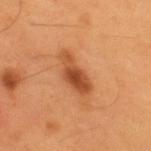Recorded during total-body skin imaging; not selected for excision or biopsy. About 5 mm across. A 15 mm close-up extracted from a 3D total-body photography capture. A male patient aged 53 to 57. The lesion-visualizer software estimated an area of roughly 8 mm² and two-axis asymmetry of about 0.3. The software also gave a lesion color around L≈48 a*≈28 b*≈39 in CIELAB, a lesion–skin lightness drop of about 13, and a normalized lesion–skin contrast near 9. It also reported a border-irregularity index near 4/10, a within-lesion color-variation index near 3/10, and peripheral color asymmetry of about 1. The tile uses cross-polarized illumination. From the upper back.